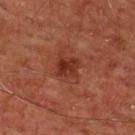* notes · no biopsy performed (imaged during a skin exam)
* lighting · cross-polarized illumination
* automated lesion analysis · an area of roughly 5.5 mm² and two-axis asymmetry of about 0.25; border irregularity of about 2.5 on a 0–10 scale and a within-lesion color-variation index near 3.5/10; lesion-presence confidence of about 100/100
* lesion size · about 3 mm
* site · the chest
* image · 15 mm crop, total-body photography
* patient · male, roughly 60 years of age